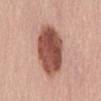| key | value |
|---|---|
| workup | catalogued during a skin exam; not biopsied |
| size | about 6.5 mm |
| patient | female, aged 63–67 |
| image | total-body-photography crop, ~15 mm field of view |
| illumination | white-light illumination |
| site | the back |